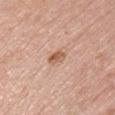| field | value |
|---|---|
| follow-up | catalogued during a skin exam; not biopsied |
| anatomic site | the chest |
| size | ~2.5 mm (longest diameter) |
| subject | female, aged around 50 |
| image | total-body-photography crop, ~15 mm field of view |
| lighting | white-light |